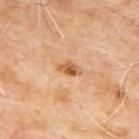– follow-up · no biopsy performed (imaged during a skin exam)
– image source · ~15 mm tile from a whole-body skin photo
– TBP lesion metrics · border irregularity of about 2.5 on a 0–10 scale, a color-variation rating of about 4.5/10, and peripheral color asymmetry of about 1.5; a classifier nevus-likeness of about 35/100 and lesion-presence confidence of about 100/100
– subject · male, approximately 65 years of age
– site · the upper back
– tile lighting · cross-polarized